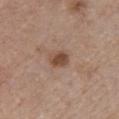Part of a total-body skin-imaging series; this lesion was reviewed on a skin check and was not flagged for biopsy. A female patient approximately 45 years of age. The lesion is located on the chest. Imaged with white-light lighting. A 15 mm close-up extracted from a 3D total-body photography capture. The lesion's longest dimension is about 2.5 mm. Automated image analysis of the tile measured a shape eccentricity near 0.7 and two-axis asymmetry of about 0.2. The software also gave a mean CIELAB color near L≈47 a*≈19 b*≈28, a lesion–skin lightness drop of about 12, and a normalized lesion–skin contrast near 9. And it measured a nevus-likeness score of about 85/100 and a detector confidence of about 100 out of 100 that the crop contains a lesion.